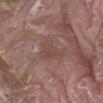{"biopsy_status": "not biopsied; imaged during a skin examination", "site": "lower back", "lighting": "white-light", "patient": {"sex": "male", "age_approx": 40}, "image": {"source": "total-body photography crop", "field_of_view_mm": 15}, "automated_metrics": {"area_mm2_approx": 8.5, "shape_asymmetry": 0.5, "color_variation_0_10": 2.0, "nevus_likeness_0_100": 0, "lesion_detection_confidence_0_100": 90}, "lesion_size": {"long_diameter_mm_approx": 4.5}}